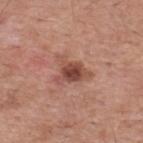Notes:
- notes — no biopsy performed (imaged during a skin exam)
- patient — male, aged approximately 55
- imaging modality — ~15 mm crop, total-body skin-cancer survey
- body site — the back